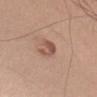{"biopsy_status": "not biopsied; imaged during a skin examination", "site": "left upper arm", "patient": {"sex": "male", "age_approx": 50}, "image": {"source": "total-body photography crop", "field_of_view_mm": 15}}